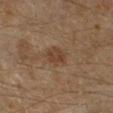The lesion was photographed on a routine skin check and not biopsied; there is no pathology result. A 15 mm close-up tile from a total-body photography series done for melanoma screening. On the right lower leg. The lesion-visualizer software estimated lesion-presence confidence of about 100/100. The patient is in their mid-50s. About 3 mm across. Imaged with cross-polarized lighting.